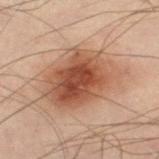Impression: Captured during whole-body skin photography for melanoma surveillance; the lesion was not biopsied. Acquisition and patient details: The lesion's longest dimension is about 7.5 mm. Cropped from a total-body skin-imaging series; the visible field is about 15 mm. From the left leg. This is a cross-polarized tile. The subject is a male aged 48 to 52. Automated image analysis of the tile measured border irregularity of about 2.5 on a 0–10 scale and a within-lesion color-variation index near 6.5/10.